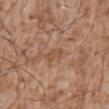| feature | finding |
|---|---|
| notes | catalogued during a skin exam; not biopsied |
| lighting | white-light illumination |
| subject | male, aged 43 to 47 |
| location | the abdomen |
| image | ~15 mm tile from a whole-body skin photo |
| lesion size | ≈2.5 mm |